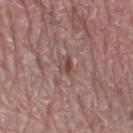biopsy status: no biopsy performed (imaged during a skin exam); body site: the right lower leg; acquisition: ~15 mm tile from a whole-body skin photo; size: ~2.5 mm (longest diameter); subject: female, approximately 65 years of age.